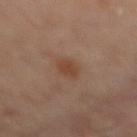biopsy status = total-body-photography surveillance lesion; no biopsy.